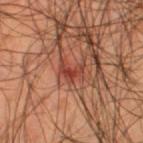Clinical impression:
Captured during whole-body skin photography for melanoma surveillance; the lesion was not biopsied.
Acquisition and patient details:
Automated tile analysis of the lesion measured a footprint of about 5.5 mm² and two-axis asymmetry of about 0.45. It also reported a border-irregularity rating of about 5.5/10, internal color variation of about 3 on a 0–10 scale, and peripheral color asymmetry of about 0.5. About 3 mm across. Cropped from a total-body skin-imaging series; the visible field is about 15 mm. The tile uses cross-polarized illumination. On the left thigh. The patient is a male roughly 55 years of age.